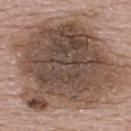biopsy_status: not biopsied; imaged during a skin examination
site: upper back
automated_metrics:
  cielab_L: 47
  cielab_a: 15
  cielab_b: 24
  border_irregularity_0_10: 4.0
  nevus_likeness_0_100: 5
  lesion_detection_confidence_0_100: 100
patient:
  sex: male
  age_approx: 55
lighting: white-light
image:
  source: total-body photography crop
  field_of_view_mm: 15